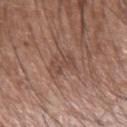Captured during whole-body skin photography for melanoma surveillance; the lesion was not biopsied.
Captured under white-light illumination.
A roughly 15 mm field-of-view crop from a total-body skin photograph.
Longest diameter approximately 3.5 mm.
On the right forearm.
A male subject roughly 55 years of age.